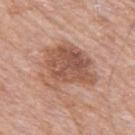workup = no biopsy performed (imaged during a skin exam); illumination = white-light; acquisition = ~15 mm crop, total-body skin-cancer survey; patient = male, roughly 80 years of age; body site = the upper back.